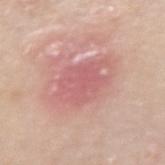Q: Is there a histopathology result?
A: catalogued during a skin exam; not biopsied
Q: How was this image acquired?
A: 15 mm crop, total-body photography
Q: How large is the lesion?
A: about 5.5 mm
Q: What is the anatomic site?
A: the arm
Q: What lighting was used for the tile?
A: white-light illumination
Q: Who is the patient?
A: female, aged around 70
Q: What did automated image analysis measure?
A: an average lesion color of about L≈60 a*≈28 b*≈22 (CIELAB), a lesion–skin lightness drop of about 8, and a normalized border contrast of about 5.5; lesion-presence confidence of about 100/100